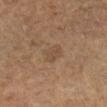Notes:
• workup: total-body-photography surveillance lesion; no biopsy
• tile lighting: cross-polarized
• site: the leg
• automated lesion analysis: a within-lesion color-variation index near 1.5/10 and radial color variation of about 0.5
• patient: female, aged 68–72
• size: about 3 mm
• imaging modality: ~15 mm tile from a whole-body skin photo